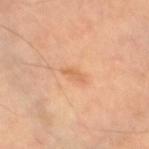| key | value |
|---|---|
| notes | catalogued during a skin exam; not biopsied |
| image | ~15 mm tile from a whole-body skin photo |
| subject | female, roughly 60 years of age |
| location | the right leg |
| diameter | ≈2.5 mm |